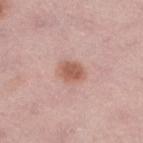follow-up = catalogued during a skin exam; not biopsied | lesion diameter = about 3 mm | image = 15 mm crop, total-body photography | lighting = white-light illumination | subject = female, aged approximately 50 | location = the left lower leg.